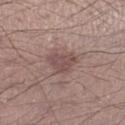notes=imaged on a skin check; not biopsied | tile lighting=white-light | anatomic site=the right lower leg | patient=male, approximately 60 years of age | imaging modality=~15 mm tile from a whole-body skin photo | automated metrics=roughly 9 lightness units darker than nearby skin and a normalized lesion–skin contrast near 6.5; a nevus-likeness score of about 0/100.